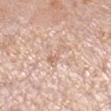Clinical impression:
Imaged during a routine full-body skin examination; the lesion was not biopsied and no histopathology is available.
Clinical summary:
Cropped from a total-body skin-imaging series; the visible field is about 15 mm. A female patient approximately 40 years of age. The lesion is on the right lower leg.